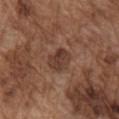Clinical impression: The lesion was tiled from a total-body skin photograph and was not biopsied. Acquisition and patient details: A male subject, about 75 years old. Located on the right upper arm. A region of skin cropped from a whole-body photographic capture, roughly 15 mm wide. This is a white-light tile. The total-body-photography lesion software estimated an average lesion color of about L≈38 a*≈19 b*≈26 (CIELAB), roughly 8 lightness units darker than nearby skin, and a lesion-to-skin contrast of about 7.5 (normalized; higher = more distinct). The analysis additionally found a color-variation rating of about 3/10 and radial color variation of about 1. It also reported a classifier nevus-likeness of about 0/100 and a detector confidence of about 100 out of 100 that the crop contains a lesion. Measured at roughly 3 mm in maximum diameter.